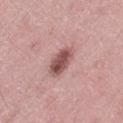<case>
  <lesion_size>
    <long_diameter_mm_approx>3.5</long_diameter_mm_approx>
  </lesion_size>
  <site>right thigh</site>
  <patient>
    <sex>male</sex>
    <age_approx>45</age_approx>
  </patient>
  <lighting>white-light</lighting>
  <image>
    <source>total-body photography crop</source>
    <field_of_view_mm>15</field_of_view_mm>
  </image>
</case>